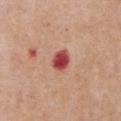Captured during whole-body skin photography for melanoma surveillance; the lesion was not biopsied.